A female subject approximately 25 years of age, from the chest, a 15 mm close-up tile from a total-body photography series done for melanoma screening.
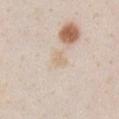Clinical summary: Captured under white-light illumination. Diagnosis: On biopsy, histopathology showed a dysplastic (Clark) nevus.